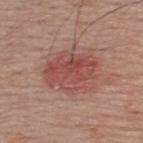Notes:
– biopsy status — catalogued during a skin exam; not biopsied
– lighting — white-light
– TBP lesion metrics — a footprint of about 20 mm², a shape eccentricity near 0.7, and two-axis asymmetry of about 0.2; a lesion–skin lightness drop of about 10
– site — the upper back
– acquisition — 15 mm crop, total-body photography
– subject — male, roughly 40 years of age
– size — ~6 mm (longest diameter)